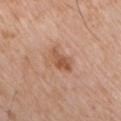<record>
  <biopsy_status>not biopsied; imaged during a skin examination</biopsy_status>
  <automated_metrics>
    <area_mm2_approx>5.5</area_mm2_approx>
    <eccentricity>0.8</eccentricity>
    <shape_asymmetry>0.3</shape_asymmetry>
    <nevus_likeness_0_100>40</nevus_likeness_0_100>
    <lesion_detection_confidence_0_100>100</lesion_detection_confidence_0_100>
  </automated_metrics>
  <patient>
    <sex>male</sex>
    <age_approx>65</age_approx>
  </patient>
  <lighting>white-light</lighting>
  <lesion_size>
    <long_diameter_mm_approx>3.5</long_diameter_mm_approx>
  </lesion_size>
  <site>chest</site>
  <image>
    <source>total-body photography crop</source>
    <field_of_view_mm>15</field_of_view_mm>
  </image>
</record>